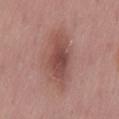lesion_size:
  long_diameter_mm_approx: 6.5
site: lower back
patient:
  sex: male
  age_approx: 55
lighting: white-light
image:
  source: total-body photography crop
  field_of_view_mm: 15